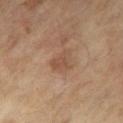Findings:
- biopsy status · catalogued during a skin exam; not biopsied
- automated lesion analysis · a lesion area of about 4.5 mm², an outline eccentricity of about 0.55 (0 = round, 1 = elongated), and a shape-asymmetry score of about 0.45 (0 = symmetric); an average lesion color of about L≈48 a*≈19 b*≈30 (CIELAB), a lesion–skin lightness drop of about 7, and a normalized border contrast of about 5.5; border irregularity of about 4.5 on a 0–10 scale, a within-lesion color-variation index near 1/10, and radial color variation of about 0.5; a classifier nevus-likeness of about 5/100 and a lesion-detection confidence of about 100/100
- image source · ~15 mm crop, total-body skin-cancer survey
- diameter · about 3 mm
- patient · male, aged around 65